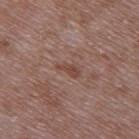The lesion was tiled from a total-body skin photograph and was not biopsied.
The lesion is located on the upper back.
A male subject, in their 50s.
This is a white-light tile.
This image is a 15 mm lesion crop taken from a total-body photograph.